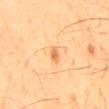| feature | finding |
|---|---|
| workup | no biopsy performed (imaged during a skin exam) |
| imaging modality | ~15 mm crop, total-body skin-cancer survey |
| lesion size | about 2 mm |
| illumination | cross-polarized illumination |
| location | the mid back |
| automated metrics | a footprint of about 2 mm², a shape eccentricity near 0.9, and two-axis asymmetry of about 0.4; roughly 11 lightness units darker than nearby skin and a normalized lesion–skin contrast near 7; an automated nevus-likeness rating near 25 out of 100 and lesion-presence confidence of about 100/100 |
| subject | male, aged around 60 |